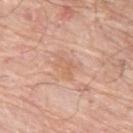The lesion was photographed on a routine skin check and not biopsied; there is no pathology result. An algorithmic analysis of the crop reported a mean CIELAB color near L≈63 a*≈22 b*≈33 and a lesion-to-skin contrast of about 4.5 (normalized; higher = more distinct). A roughly 15 mm field-of-view crop from a total-body skin photograph. From the left thigh. Captured under white-light illumination. The patient is a male approximately 80 years of age. Approximately 2.5 mm at its widest.